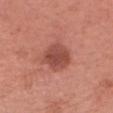Q: What kind of image is this?
A: 15 mm crop, total-body photography
Q: Where on the body is the lesion?
A: the head or neck
Q: How was the tile lit?
A: white-light
Q: What is the lesion's diameter?
A: about 4 mm
Q: Who is the patient?
A: female, aged 63 to 67